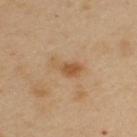Assessment: Captured during whole-body skin photography for melanoma surveillance; the lesion was not biopsied. Image and clinical context: Located on the left upper arm. A female patient, aged 38 to 42. Imaged with cross-polarized lighting. About 3.5 mm across. This image is a 15 mm lesion crop taken from a total-body photograph.